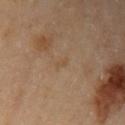workup — no biopsy performed (imaged during a skin exam)
site — the left upper arm
subject — male, aged around 85 or older
acquisition — ~15 mm crop, total-body skin-cancer survey